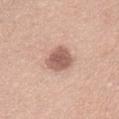Notes:
- workup · no biopsy performed (imaged during a skin exam)
- illumination · white-light
- lesion size · about 3.5 mm
- patient · male, approximately 55 years of age
- site · the chest
- image source · total-body-photography crop, ~15 mm field of view